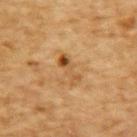follow-up: catalogued during a skin exam; not biopsied
acquisition: total-body-photography crop, ~15 mm field of view
subject: male, aged 83 or over
lesion diameter: about 4 mm
site: the upper back